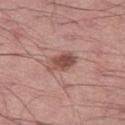A male patient, in their mid- to late 40s. From the right thigh. This image is a 15 mm lesion crop taken from a total-body photograph.